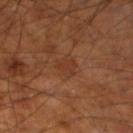notes=catalogued during a skin exam; not biopsied | subject=male, approximately 60 years of age | site=the left lower leg | size=about 3 mm | image source=total-body-photography crop, ~15 mm field of view | lighting=cross-polarized illumination.